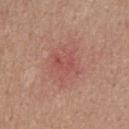Q: Is there a histopathology result?
A: total-body-photography surveillance lesion; no biopsy
Q: What is the imaging modality?
A: ~15 mm crop, total-body skin-cancer survey
Q: What is the anatomic site?
A: the upper back
Q: Patient demographics?
A: female, about 40 years old